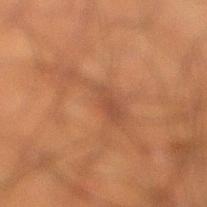Findings:
– workup: imaged on a skin check; not biopsied
– patient: male, in their 50s
– lighting: cross-polarized illumination
– image-analysis metrics: a lesion color around L≈39 a*≈19 b*≈28 in CIELAB and a normalized lesion–skin contrast near 5; a border-irregularity rating of about 10/10 and radial color variation of about 0.5
– body site: the right lower leg
– image source: 15 mm crop, total-body photography